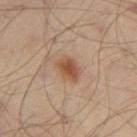biopsy status: catalogued during a skin exam; not biopsied | site: the left thigh | image: ~15 mm crop, total-body skin-cancer survey | image-analysis metrics: a lesion area of about 6 mm² and a shape-asymmetry score of about 0.2 (0 = symmetric); a mean CIELAB color near L≈52 a*≈20 b*≈32, a lesion–skin lightness drop of about 10, and a normalized lesion–skin contrast near 8; a border-irregularity rating of about 2/10, internal color variation of about 4 on a 0–10 scale, and a peripheral color-asymmetry measure near 1; an automated nevus-likeness rating near 90 out of 100 and a lesion-detection confidence of about 100/100 | lesion size: ≈3 mm | patient: male, approximately 55 years of age | illumination: cross-polarized.